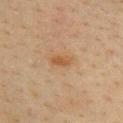Part of a total-body skin-imaging series; this lesion was reviewed on a skin check and was not flagged for biopsy.
A region of skin cropped from a whole-body photographic capture, roughly 15 mm wide.
On the chest.
The patient is a female aged 38–42.